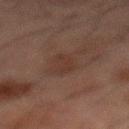{"biopsy_status": "not biopsied; imaged during a skin examination", "lighting": "cross-polarized", "image": {"source": "total-body photography crop", "field_of_view_mm": 15}, "site": "mid back", "lesion_size": {"long_diameter_mm_approx": 3.5}, "patient": {"sex": "male", "age_approx": 50}}